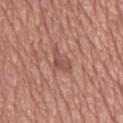biopsy status — imaged on a skin check; not biopsied | automated lesion analysis — a footprint of about 5.5 mm², an eccentricity of roughly 0.85, and two-axis asymmetry of about 0.45; an average lesion color of about L≈52 a*≈24 b*≈27 (CIELAB), roughly 7 lightness units darker than nearby skin, and a normalized lesion–skin contrast near 5.5; an automated nevus-likeness rating near 0 out of 100 and lesion-presence confidence of about 85/100 | tile lighting — white-light illumination | lesion size — ~4 mm (longest diameter) | imaging modality — 15 mm crop, total-body photography | location — the front of the torso | patient — male, in their mid-70s.